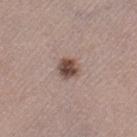Clinical impression: Imaged during a routine full-body skin examination; the lesion was not biopsied and no histopathology is available. Clinical summary: Captured under white-light illumination. A female subject, aged around 50. The lesion is on the left thigh. An algorithmic analysis of the crop reported a lesion color around L≈47 a*≈17 b*≈22 in CIELAB. The analysis additionally found a border-irregularity index near 1.5/10, internal color variation of about 4 on a 0–10 scale, and radial color variation of about 1.5. It also reported a nevus-likeness score of about 95/100. Cropped from a total-body skin-imaging series; the visible field is about 15 mm.